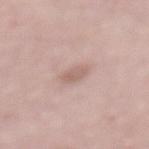Q: Is there a histopathology result?
A: total-body-photography surveillance lesion; no biopsy
Q: Where on the body is the lesion?
A: the mid back
Q: Who is the patient?
A: female, aged 63–67
Q: What lighting was used for the tile?
A: white-light
Q: What is the imaging modality?
A: ~15 mm crop, total-body skin-cancer survey
Q: Lesion size?
A: about 3 mm